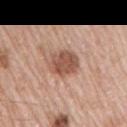The total-body-photography lesion software estimated a footprint of about 9.5 mm², an eccentricity of roughly 0.55, and a symmetry-axis asymmetry near 0.15. It also reported a nevus-likeness score of about 40/100 and a lesion-detection confidence of about 100/100. On the right upper arm. A male subject, about 65 years old. Cropped from a whole-body photographic skin survey; the tile spans about 15 mm. About 4 mm across. Captured under white-light illumination.